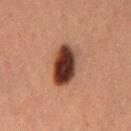| feature | finding |
|---|---|
| follow-up | total-body-photography surveillance lesion; no biopsy |